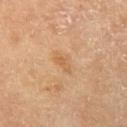Clinical summary: Cropped from a whole-body photographic skin survey; the tile spans about 15 mm. The recorded lesion diameter is about 2.5 mm. On the left upper arm. The tile uses cross-polarized illumination. A female patient aged 63 to 67.